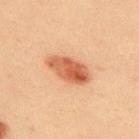{
  "image": {
    "source": "total-body photography crop",
    "field_of_view_mm": 15
  },
  "patient": {
    "sex": "male",
    "age_approx": 60
  },
  "lighting": "cross-polarized",
  "automated_metrics": {
    "cielab_L": 49,
    "cielab_a": 24,
    "cielab_b": 32,
    "vs_skin_darker_L": 12.0,
    "vs_skin_contrast_norm": 9.0,
    "border_irregularity_0_10": 2.0,
    "color_variation_0_10": 6.5,
    "peripheral_color_asymmetry": 2.5,
    "nevus_likeness_0_100": 100,
    "lesion_detection_confidence_0_100": 100
  },
  "site": "back"
}